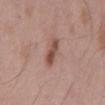Located on the mid back.
Cropped from a whole-body photographic skin survey; the tile spans about 15 mm.
The recorded lesion diameter is about 4 mm.
A male subject, aged around 55.
The lesion-visualizer software estimated a lesion color around L≈51 a*≈21 b*≈25 in CIELAB, a lesion–skin lightness drop of about 11, and a normalized border contrast of about 8. The software also gave a nevus-likeness score of about 60/100 and lesion-presence confidence of about 100/100.
Imaged with white-light lighting.